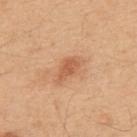Captured during whole-body skin photography for melanoma surveillance; the lesion was not biopsied. Located on the upper back. A male subject, in their 50s. Cropped from a total-body skin-imaging series; the visible field is about 15 mm.